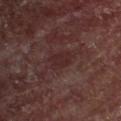The lesion was photographed on a routine skin check and not biopsied; there is no pathology result.
Cropped from a whole-body photographic skin survey; the tile spans about 15 mm.
Captured under cross-polarized illumination.
Longest diameter approximately 3 mm.
The lesion is located on the front of the torso.
The patient is a male approximately 55 years of age.